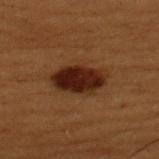• workup: total-body-photography surveillance lesion; no biopsy
• lesion size: ~5.5 mm (longest diameter)
• imaging modality: total-body-photography crop, ~15 mm field of view
• location: the upper back
• tile lighting: cross-polarized
• patient: male, aged 48 to 52
• automated lesion analysis: an area of roughly 14 mm² and a symmetry-axis asymmetry near 0.15; a border-irregularity rating of about 1.5/10, internal color variation of about 5 on a 0–10 scale, and radial color variation of about 1.5; a nevus-likeness score of about 95/100